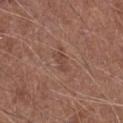{
  "biopsy_status": "not biopsied; imaged during a skin examination",
  "lighting": "white-light",
  "site": "leg",
  "image": {
    "source": "total-body photography crop",
    "field_of_view_mm": 15
  },
  "lesion_size": {
    "long_diameter_mm_approx": 2.5
  },
  "patient": {
    "sex": "male",
    "age_approx": 75
  },
  "automated_metrics": {
    "border_irregularity_0_10": 3.5,
    "color_variation_0_10": 0.5,
    "peripheral_color_asymmetry": 0.0,
    "nevus_likeness_0_100": 0,
    "lesion_detection_confidence_0_100": 100
  }
}